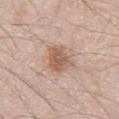No biopsy was performed on this lesion — it was imaged during a full skin examination and was not determined to be concerning. Located on the abdomen. The tile uses white-light illumination. Longest diameter approximately 4.5 mm. A 15 mm crop from a total-body photograph taken for skin-cancer surveillance. A male patient, aged 43–47.